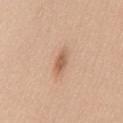{"biopsy_status": "not biopsied; imaged during a skin examination", "patient": {"sex": "female", "age_approx": 40}, "site": "chest", "image": {"source": "total-body photography crop", "field_of_view_mm": 15}}